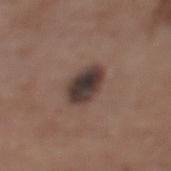Part of a total-body skin-imaging series; this lesion was reviewed on a skin check and was not flagged for biopsy. The lesion-visualizer software estimated a footprint of about 9.5 mm² and a shape eccentricity near 0.7. It also reported a mean CIELAB color near L≈36 a*≈14 b*≈18 and roughly 14 lightness units darker than nearby skin. It also reported a within-lesion color-variation index near 5.5/10 and peripheral color asymmetry of about 1.5. Imaged with white-light lighting. This image is a 15 mm lesion crop taken from a total-body photograph. The lesion is on the leg. The patient is a male about 65 years old.